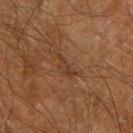follow-up = total-body-photography surveillance lesion; no biopsy | patient = male, aged 58 to 62 | body site = the leg | acquisition = total-body-photography crop, ~15 mm field of view.